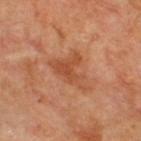Impression: Part of a total-body skin-imaging series; this lesion was reviewed on a skin check and was not flagged for biopsy. Acquisition and patient details: The lesion's longest dimension is about 5 mm. A region of skin cropped from a whole-body photographic capture, roughly 15 mm wide. The patient is a male in their 70s. From the back. Captured under cross-polarized illumination.